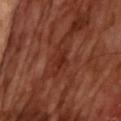{
  "lesion_size": {
    "long_diameter_mm_approx": 3.5
  },
  "image": {
    "source": "total-body photography crop",
    "field_of_view_mm": 15
  },
  "automated_metrics": {
    "area_mm2_approx": 4.5
  },
  "patient": {
    "sex": "male",
    "age_approx": 65
  }
}